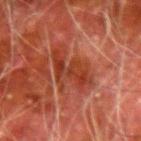No biopsy was performed on this lesion — it was imaged during a full skin examination and was not determined to be concerning. The subject is a male aged 78–82. The recorded lesion diameter is about 6.5 mm. The lesion is on the right forearm. Automated image analysis of the tile measured an eccentricity of roughly 0.9 and a symmetry-axis asymmetry near 0.4. The software also gave a mean CIELAB color near L≈32 a*≈27 b*≈28, a lesion–skin lightness drop of about 7, and a normalized lesion–skin contrast near 7.5. A close-up tile cropped from a whole-body skin photograph, about 15 mm across.